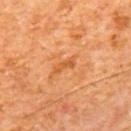Part of a total-body skin-imaging series; this lesion was reviewed on a skin check and was not flagged for biopsy.
The lesion is located on the mid back.
The total-body-photography lesion software estimated border irregularity of about 4 on a 0–10 scale and peripheral color asymmetry of about 0. The software also gave an automated nevus-likeness rating near 0 out of 100 and a detector confidence of about 100 out of 100 that the crop contains a lesion.
A male subject about 65 years old.
The lesion's longest dimension is about 2.5 mm.
A close-up tile cropped from a whole-body skin photograph, about 15 mm across.
Imaged with cross-polarized lighting.